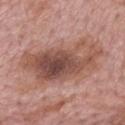Recorded during total-body skin imaging; not selected for excision or biopsy. A region of skin cropped from a whole-body photographic capture, roughly 15 mm wide. Approximately 10 mm at its widest. The lesion is located on the mid back. The lesion-visualizer software estimated a lesion color around L≈50 a*≈22 b*≈26 in CIELAB, roughly 12 lightness units darker than nearby skin, and a normalized lesion–skin contrast near 8.5. The analysis additionally found an automated nevus-likeness rating near 45 out of 100 and a detector confidence of about 100 out of 100 that the crop contains a lesion. A male patient, about 75 years old.